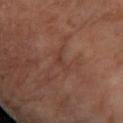Captured during whole-body skin photography for melanoma surveillance; the lesion was not biopsied. A roughly 15 mm field-of-view crop from a total-body skin photograph. The lesion is on the left forearm. A female subject, aged 68–72. Automated image analysis of the tile measured a lesion color around L≈39 a*≈22 b*≈27 in CIELAB, roughly 5 lightness units darker than nearby skin, and a normalized lesion–skin contrast near 5. And it measured a border-irregularity rating of about 6/10, internal color variation of about 0 on a 0–10 scale, and a peripheral color-asymmetry measure near 0. And it measured a classifier nevus-likeness of about 0/100 and a lesion-detection confidence of about 70/100.